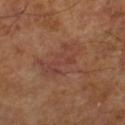Impression:
No biopsy was performed on this lesion — it was imaged during a full skin examination and was not determined to be concerning.
Acquisition and patient details:
A male patient in their mid-60s. This image is a 15 mm lesion crop taken from a total-body photograph.